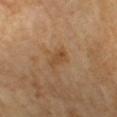Captured during whole-body skin photography for melanoma surveillance; the lesion was not biopsied.
Longest diameter approximately 3 mm.
The lesion is on the left forearm.
A female subject, aged 68 to 72.
A 15 mm close-up extracted from a 3D total-body photography capture.
Imaged with cross-polarized lighting.